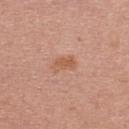  biopsy_status: not biopsied; imaged during a skin examination
  site: upper back
  lesion_size:
    long_diameter_mm_approx: 3.0
  patient:
    sex: female
    age_approx: 40
  lighting: white-light
  image:
    source: total-body photography crop
    field_of_view_mm: 15